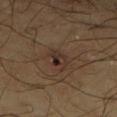The lesion was photographed on a routine skin check and not biopsied; there is no pathology result. The lesion is on the right lower leg. A male patient, about 65 years old. A lesion tile, about 15 mm wide, cut from a 3D total-body photograph.